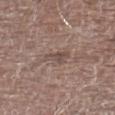* workup — catalogued during a skin exam; not biopsied
* image — 15 mm crop, total-body photography
* automated metrics — an outline eccentricity of about 0.8 (0 = round, 1 = elongated); a border-irregularity rating of about 4/10, a color-variation rating of about 1.5/10, and peripheral color asymmetry of about 0.5; a nevus-likeness score of about 0/100
* patient — male, approximately 75 years of age
* anatomic site — the left lower leg